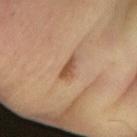<case>
<biopsy_status>not biopsied; imaged during a skin examination</biopsy_status>
<image>
  <source>total-body photography crop</source>
  <field_of_view_mm>15</field_of_view_mm>
</image>
<site>arm</site>
<patient>
  <sex>male</sex>
  <age_approx>65</age_approx>
</patient>
</case>